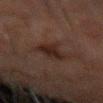Q: Was this lesion biopsied?
A: total-body-photography surveillance lesion; no biopsy
Q: What is the anatomic site?
A: the left forearm
Q: What is the imaging modality?
A: total-body-photography crop, ~15 mm field of view
Q: Illumination type?
A: cross-polarized
Q: Patient demographics?
A: male, aged 58–62
Q: Lesion size?
A: ≈4 mm